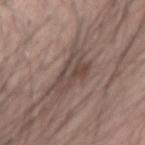<record>
<biopsy_status>not biopsied; imaged during a skin examination</biopsy_status>
<site>right forearm</site>
<patient>
  <sex>male</sex>
  <age_approx>25</age_approx>
</patient>
<lesion_size>
  <long_diameter_mm_approx>5.0</long_diameter_mm_approx>
</lesion_size>
<lighting>white-light</lighting>
<image>
  <source>total-body photography crop</source>
  <field_of_view_mm>15</field_of_view_mm>
</image>
</record>